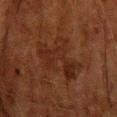Clinical impression:
Recorded during total-body skin imaging; not selected for excision or biopsy.
Background:
Captured under cross-polarized illumination. Cropped from a total-body skin-imaging series; the visible field is about 15 mm. The lesion is located on the head or neck. A male subject about 65 years old. The recorded lesion diameter is about 6 mm. The total-body-photography lesion software estimated a lesion area of about 18 mm², an eccentricity of roughly 0.6, and a shape-asymmetry score of about 0.5 (0 = symmetric). It also reported a lesion–skin lightness drop of about 5 and a normalized border contrast of about 6. And it measured a border-irregularity index near 7/10, a within-lesion color-variation index near 4/10, and a peripheral color-asymmetry measure near 1.5. And it measured a nevus-likeness score of about 0/100 and a detector confidence of about 100 out of 100 that the crop contains a lesion.